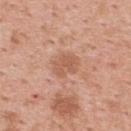{
  "biopsy_status": "not biopsied; imaged during a skin examination",
  "patient": {
    "sex": "female",
    "age_approx": 50
  },
  "image": {
    "source": "total-body photography crop",
    "field_of_view_mm": 15
  },
  "site": "upper back"
}